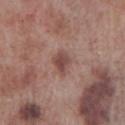biopsy status: total-body-photography surveillance lesion; no biopsy
illumination: white-light illumination
automated lesion analysis: a lesion color around L≈46 a*≈21 b*≈22 in CIELAB, about 10 CIELAB-L* units darker than the surrounding skin, and a lesion-to-skin contrast of about 8 (normalized; higher = more distinct); a border-irregularity index near 2.5/10 and radial color variation of about 1
lesion diameter: about 2.5 mm
body site: the right lower leg
patient: male, approximately 70 years of age
acquisition: ~15 mm tile from a whole-body skin photo